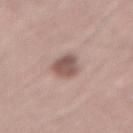No biopsy was performed on this lesion — it was imaged during a full skin examination and was not determined to be concerning.
A close-up tile cropped from a whole-body skin photograph, about 15 mm across.
Automated image analysis of the tile measured a footprint of about 7 mm², an outline eccentricity of about 0.8 (0 = round, 1 = elongated), and a symmetry-axis asymmetry near 0.25. The analysis additionally found a lesion color around L≈54 a*≈18 b*≈22 in CIELAB and a normalized lesion–skin contrast near 8.5. The software also gave a border-irregularity index near 2.5/10 and radial color variation of about 1.
A male patient in their mid-50s.